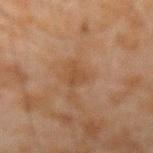Clinical impression: Part of a total-body skin-imaging series; this lesion was reviewed on a skin check and was not flagged for biopsy. Image and clinical context: Imaged with cross-polarized lighting. A 15 mm close-up tile from a total-body photography series done for melanoma screening. The total-body-photography lesion software estimated a mean CIELAB color near L≈38 a*≈17 b*≈29, about 6 CIELAB-L* units darker than the surrounding skin, and a normalized border contrast of about 5.5. The software also gave a color-variation rating of about 0.5/10 and a peripheral color-asymmetry measure near 0. The analysis additionally found a classifier nevus-likeness of about 0/100 and a lesion-detection confidence of about 100/100. A male subject aged around 45. From the right forearm.